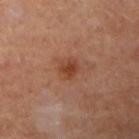Assessment:
The lesion was tiled from a total-body skin photograph and was not biopsied.